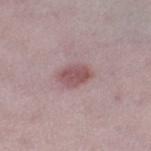biopsy status = total-body-photography surveillance lesion; no biopsy
image = ~15 mm tile from a whole-body skin photo
location = the leg
patient = female, roughly 45 years of age